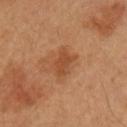Q: Was a biopsy performed?
A: total-body-photography surveillance lesion; no biopsy
Q: What is the anatomic site?
A: the left upper arm
Q: What is the imaging modality?
A: ~15 mm tile from a whole-body skin photo
Q: Illumination type?
A: cross-polarized illumination
Q: Lesion size?
A: about 3.5 mm
Q: What did automated image analysis measure?
A: a lesion area of about 6 mm², a shape eccentricity near 0.75, and a shape-asymmetry score of about 0.3 (0 = symmetric)
Q: Patient demographics?
A: male, in their mid-50s